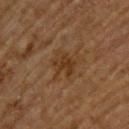Notes:
* illumination · cross-polarized illumination
* image source · ~15 mm tile from a whole-body skin photo
* location · the upper back
* lesion size · ≈3.5 mm
* automated metrics · an average lesion color of about L≈32 a*≈17 b*≈29 (CIELAB); a nevus-likeness score of about 0/100 and a detector confidence of about 100 out of 100 that the crop contains a lesion
* subject · male, approximately 65 years of age